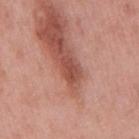Notes:
– workup: no biopsy performed (imaged during a skin exam)
– location: the mid back
– subject: male, aged 53–57
– lesion size: about 3 mm
– automated lesion analysis: a mean CIELAB color near L≈48 a*≈28 b*≈28 and a normalized lesion–skin contrast near 8; a classifier nevus-likeness of about 0/100
– image source: 15 mm crop, total-body photography
– illumination: white-light illumination